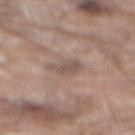{
  "patient": {
    "sex": "male",
    "age_approx": 80
  },
  "lighting": "white-light",
  "site": "mid back",
  "image": {
    "source": "total-body photography crop",
    "field_of_view_mm": 15
  },
  "automated_metrics": {
    "area_mm2_approx": 4.5,
    "eccentricity": 0.6,
    "shape_asymmetry": 0.3,
    "nevus_likeness_0_100": 0
  }
}